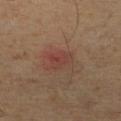Imaged during a routine full-body skin examination; the lesion was not biopsied and no histopathology is available. A roughly 15 mm field-of-view crop from a total-body skin photograph. The lesion's longest dimension is about 2.5 mm. A male patient aged 53–57. The lesion is located on the right lower leg. Imaged with cross-polarized lighting.